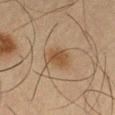| field | value |
|---|---|
| subject | male, roughly 65 years of age |
| image source | ~15 mm tile from a whole-body skin photo |
| location | the left thigh |
| illumination | cross-polarized illumination |
| lesion size | about 3.5 mm |
| image-analysis metrics | a footprint of about 7.5 mm² and an eccentricity of roughly 0.6; an average lesion color of about L≈41 a*≈14 b*≈29 (CIELAB), a lesion–skin lightness drop of about 7, and a lesion-to-skin contrast of about 7 (normalized; higher = more distinct); a border-irregularity index near 2.5/10, a color-variation rating of about 2.5/10, and peripheral color asymmetry of about 0.5; an automated nevus-likeness rating near 65 out of 100 and a detector confidence of about 100 out of 100 that the crop contains a lesion |